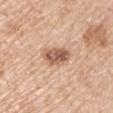This lesion was catalogued during total-body skin photography and was not selected for biopsy. A male subject approximately 70 years of age. Located on the right upper arm. Imaged with white-light lighting. A 15 mm close-up extracted from a 3D total-body photography capture.